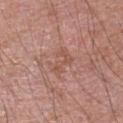No biopsy was performed on this lesion — it was imaged during a full skin examination and was not determined to be concerning.
The lesion is on the right upper arm.
A close-up tile cropped from a whole-body skin photograph, about 15 mm across.
A male patient aged approximately 50.